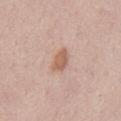Q: Was a biopsy performed?
A: no biopsy performed (imaged during a skin exam)
Q: Who is the patient?
A: male, approximately 50 years of age
Q: Lesion location?
A: the abdomen
Q: How was this image acquired?
A: ~15 mm crop, total-body skin-cancer survey
Q: How large is the lesion?
A: ≈3 mm
Q: How was the tile lit?
A: white-light illumination
Q: Automated lesion metrics?
A: a shape eccentricity near 0.8; an average lesion color of about L≈60 a*≈20 b*≈29 (CIELAB), roughly 10 lightness units darker than nearby skin, and a normalized lesion–skin contrast near 7.5; a classifier nevus-likeness of about 65/100